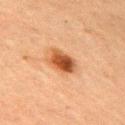No biopsy was performed on this lesion — it was imaged during a full skin examination and was not determined to be concerning. This is a cross-polarized tile. The subject is a female about 60 years old. A 15 mm crop from a total-body photograph taken for skin-cancer surveillance. The lesion is on the arm. The lesion's longest dimension is about 3.5 mm.